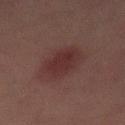Q: Was this lesion biopsied?
A: imaged on a skin check; not biopsied
Q: Lesion location?
A: the mid back
Q: Illumination type?
A: cross-polarized
Q: What is the imaging modality?
A: total-body-photography crop, ~15 mm field of view
Q: Lesion size?
A: ≈5.5 mm
Q: Automated lesion metrics?
A: a footprint of about 11 mm² and two-axis asymmetry of about 0.2; a lesion–skin lightness drop of about 6 and a normalized lesion–skin contrast near 7.5; a classifier nevus-likeness of about 95/100
Q: What are the patient's age and sex?
A: male, aged approximately 45